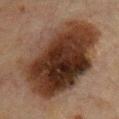Part of a total-body skin-imaging series; this lesion was reviewed on a skin check and was not flagged for biopsy.
Approximately 12 mm at its widest.
Located on the upper back.
Automated image analysis of the tile measured an area of roughly 65 mm² and a shape eccentricity near 0.8. The software also gave a within-lesion color-variation index near 8.5/10. And it measured an automated nevus-likeness rating near 25 out of 100.
The tile uses cross-polarized illumination.
A roughly 15 mm field-of-view crop from a total-body skin photograph.
A male subject aged around 65.